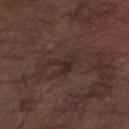Recorded during total-body skin imaging; not selected for excision or biopsy. The lesion is on the left forearm. The patient is a male approximately 75 years of age. A 15 mm close-up extracted from a 3D total-body photography capture.